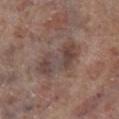This lesion was catalogued during total-body skin photography and was not selected for biopsy. A lesion tile, about 15 mm wide, cut from a 3D total-body photograph. The subject is a male roughly 70 years of age. Automated tile analysis of the lesion measured a footprint of about 16 mm², a shape eccentricity near 0.85, and a shape-asymmetry score of about 0.25 (0 = symmetric). The software also gave an average lesion color of about L≈44 a*≈15 b*≈20 (CIELAB), a lesion–skin lightness drop of about 8, and a lesion-to-skin contrast of about 6.5 (normalized; higher = more distinct). Measured at roughly 6 mm in maximum diameter. The lesion is located on the right lower leg. The tile uses white-light illumination.